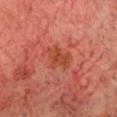Captured during whole-body skin photography for melanoma surveillance; the lesion was not biopsied.
Captured under cross-polarized illumination.
The lesion is on the head or neck.
Cropped from a total-body skin-imaging series; the visible field is about 15 mm.
Measured at roughly 4 mm in maximum diameter.
A male subject, in their 60s.
An algorithmic analysis of the crop reported a footprint of about 6 mm², a shape eccentricity near 0.85, and two-axis asymmetry of about 0.4.